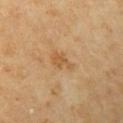Impression:
The lesion was tiled from a total-body skin photograph and was not biopsied.